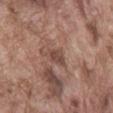imaging modality = ~15 mm tile from a whole-body skin photo | tile lighting = white-light illumination | site = the abdomen | patient = male, roughly 75 years of age.